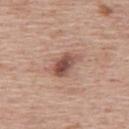| feature | finding |
|---|---|
| biopsy status | no biopsy performed (imaged during a skin exam) |
| image | total-body-photography crop, ~15 mm field of view |
| subject | male, in their 60s |
| location | the upper back |
| TBP lesion metrics | an eccentricity of roughly 0.8 and a symmetry-axis asymmetry near 0.3; a lesion–skin lightness drop of about 12 and a normalized lesion–skin contrast near 8.5; a classifier nevus-likeness of about 65/100 and a detector confidence of about 100 out of 100 that the crop contains a lesion |
| size | ~4 mm (longest diameter) |
| tile lighting | white-light illumination |